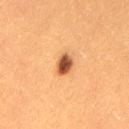This lesion was catalogued during total-body skin photography and was not selected for biopsy.
A roughly 15 mm field-of-view crop from a total-body skin photograph.
Located on the left thigh.
The subject is a female approximately 40 years of age.